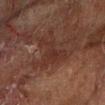  biopsy_status: not biopsied; imaged during a skin examination
  image:
    source: total-body photography crop
    field_of_view_mm: 15
  lighting: cross-polarized
  lesion_size:
    long_diameter_mm_approx: 6.0
  site: left forearm
  patient:
    sex: male
    age_approx: 60